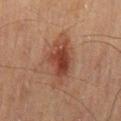Q: Is there a histopathology result?
A: catalogued during a skin exam; not biopsied
Q: Lesion size?
A: ~7 mm (longest diameter)
Q: Illumination type?
A: cross-polarized
Q: Lesion location?
A: the mid back
Q: Automated lesion metrics?
A: an area of roughly 16 mm², a shape eccentricity near 0.9, and a shape-asymmetry score of about 0.3 (0 = symmetric); an average lesion color of about L≈36 a*≈20 b*≈25 (CIELAB); a within-lesion color-variation index near 5/10 and radial color variation of about 1.5; a lesion-detection confidence of about 100/100
Q: What are the patient's age and sex?
A: male, in their 70s
Q: What is the imaging modality?
A: 15 mm crop, total-body photography